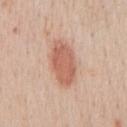Captured during whole-body skin photography for melanoma surveillance; the lesion was not biopsied. A 15 mm crop from a total-body photograph taken for skin-cancer surveillance. A male patient, about 60 years old. On the front of the torso. The recorded lesion diameter is about 5.5 mm. Imaged with white-light lighting.